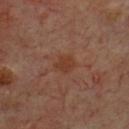Part of a total-body skin-imaging series; this lesion was reviewed on a skin check and was not flagged for biopsy.
Approximately 2.5 mm at its widest.
The subject is a male approximately 70 years of age.
Imaged with cross-polarized lighting.
The lesion is on the chest.
A 15 mm close-up extracted from a 3D total-body photography capture.